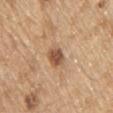Context:
A 15 mm close-up extracted from a 3D total-body photography capture. The lesion is located on the chest. Automated tile analysis of the lesion measured a mean CIELAB color near L≈53 a*≈21 b*≈32 and a lesion-to-skin contrast of about 9 (normalized; higher = more distinct). A male subject approximately 70 years of age. The recorded lesion diameter is about 2.5 mm. The tile uses white-light illumination.